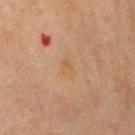This lesion was catalogued during total-body skin photography and was not selected for biopsy. A 15 mm close-up extracted from a 3D total-body photography capture. The patient is a male approximately 70 years of age. The lesion's longest dimension is about 3 mm. On the mid back.